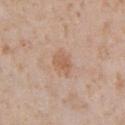Clinical impression: Imaged during a routine full-body skin examination; the lesion was not biopsied and no histopathology is available. Background: A lesion tile, about 15 mm wide, cut from a 3D total-body photograph. A male patient approximately 65 years of age. The tile uses white-light illumination. Longest diameter approximately 3 mm. The lesion is located on the chest.